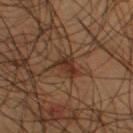biopsy status = imaged on a skin check; not biopsied
patient = male, aged around 55
image source = total-body-photography crop, ~15 mm field of view
site = the right thigh